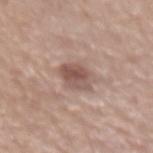Impression:
Part of a total-body skin-imaging series; this lesion was reviewed on a skin check and was not flagged for biopsy.
Background:
Imaged with white-light lighting. This image is a 15 mm lesion crop taken from a total-body photograph. A male patient aged approximately 65. On the left forearm. The lesion-visualizer software estimated a border-irregularity rating of about 2/10 and radial color variation of about 2.